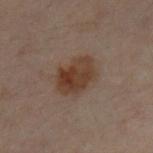Case summary:
- workup — imaged on a skin check; not biopsied
- image-analysis metrics — an eccentricity of roughly 0.65; a lesion color around L≈30 a*≈14 b*≈22 in CIELAB and roughly 8 lightness units darker than nearby skin; a border-irregularity rating of about 2/10 and radial color variation of about 1.5; a classifier nevus-likeness of about 95/100 and lesion-presence confidence of about 100/100
- body site — the chest
- patient — male, aged around 30
- illumination — cross-polarized illumination
- imaging modality — total-body-photography crop, ~15 mm field of view